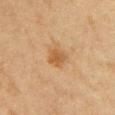  site: right upper arm
  lighting: cross-polarized
  image:
    source: total-body photography crop
    field_of_view_mm: 15
  lesion_size:
    long_diameter_mm_approx: 2.5
  patient:
    sex: female
    age_approx: 45